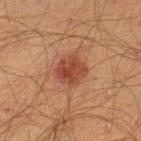The lesion was tiled from a total-body skin photograph and was not biopsied. On the left lower leg. Measured at roughly 3.5 mm in maximum diameter. A male subject aged around 45. Cropped from a whole-body photographic skin survey; the tile spans about 15 mm.